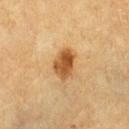A 15 mm close-up tile from a total-body photography series done for melanoma screening.
The lesion is on the left thigh.
The subject is a female about 55 years old.